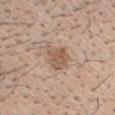Impression:
Imaged during a routine full-body skin examination; the lesion was not biopsied and no histopathology is available.
Acquisition and patient details:
Automated tile analysis of the lesion measured border irregularity of about 2.5 on a 0–10 scale. The software also gave lesion-presence confidence of about 100/100. From the head or neck. Captured under white-light illumination. A close-up tile cropped from a whole-body skin photograph, about 15 mm across. The subject is a male aged 28 to 32.